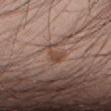Recorded during total-body skin imaging; not selected for excision or biopsy. This image is a 15 mm lesion crop taken from a total-body photograph. The subject is a male aged around 35. The lesion is on the lower back. The lesion's longest dimension is about 3.5 mm. The tile uses white-light illumination.